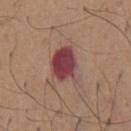This lesion was catalogued during total-body skin photography and was not selected for biopsy. A male patient in their mid- to late 60s. Measured at roughly 4.5 mm in maximum diameter. Located on the chest. A region of skin cropped from a whole-body photographic capture, roughly 15 mm wide. An algorithmic analysis of the crop reported a shape eccentricity near 0.6 and two-axis asymmetry of about 0.25. And it measured a border-irregularity rating of about 2.5/10, a within-lesion color-variation index near 5/10, and a peripheral color-asymmetry measure near 1.5.